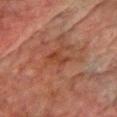| feature | finding |
|---|---|
| notes | catalogued during a skin exam; not biopsied |
| body site | the chest |
| subject | male, in their mid-70s |
| acquisition | ~15 mm tile from a whole-body skin photo |